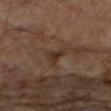follow-up: no biopsy performed (imaged during a skin exam) | patient: male, aged 83 or over | illumination: cross-polarized illumination | automated lesion analysis: a lesion area of about 4 mm² and an outline eccentricity of about 0.7 (0 = round, 1 = elongated); an average lesion color of about L≈31 a*≈16 b*≈24 (CIELAB), about 7 CIELAB-L* units darker than the surrounding skin, and a lesion-to-skin contrast of about 7 (normalized; higher = more distinct); a border-irregularity rating of about 5.5/10, a color-variation rating of about 1.5/10, and radial color variation of about 0.5; an automated nevus-likeness rating near 0 out of 100 and a lesion-detection confidence of about 95/100 | acquisition: total-body-photography crop, ~15 mm field of view | size: ~3 mm (longest diameter) | site: the right forearm.